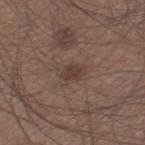Assessment: Imaged during a routine full-body skin examination; the lesion was not biopsied and no histopathology is available. Image and clinical context: The patient is a male roughly 45 years of age. A 15 mm crop from a total-body photograph taken for skin-cancer surveillance. The lesion is located on the left thigh. The lesion-visualizer software estimated a classifier nevus-likeness of about 40/100 and a lesion-detection confidence of about 100/100. About 2.5 mm across.